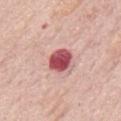{
  "biopsy_status": "not biopsied; imaged during a skin examination",
  "lighting": "white-light",
  "lesion_size": {
    "long_diameter_mm_approx": 3.0
  },
  "image": {
    "source": "total-body photography crop",
    "field_of_view_mm": 15
  },
  "patient": {
    "sex": "male",
    "age_approx": 75
  },
  "site": "mid back"
}